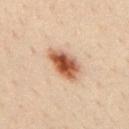Q: Was this lesion biopsied?
A: imaged on a skin check; not biopsied
Q: Patient demographics?
A: male, roughly 35 years of age
Q: How was the tile lit?
A: cross-polarized illumination
Q: Lesion location?
A: the upper back
Q: Automated lesion metrics?
A: an outline eccentricity of about 0.8 (0 = round, 1 = elongated) and a shape-asymmetry score of about 0.2 (0 = symmetric); a border-irregularity index near 2.5/10 and peripheral color asymmetry of about 2; lesion-presence confidence of about 100/100
Q: How large is the lesion?
A: about 5 mm
Q: How was this image acquired?
A: ~15 mm tile from a whole-body skin photo